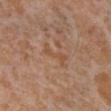The lesion is located on the leg. A female patient aged 53 to 57. Cropped from a whole-body photographic skin survey; the tile spans about 15 mm. Automated tile analysis of the lesion measured a lesion area of about 4 mm², a shape eccentricity near 0.9, and a shape-asymmetry score of about 0.4 (0 = symmetric). The software also gave a mean CIELAB color near L≈51 a*≈20 b*≈33, a lesion–skin lightness drop of about 5, and a normalized lesion–skin contrast near 5.5. It also reported border irregularity of about 6 on a 0–10 scale and radial color variation of about 0. The analysis additionally found a nevus-likeness score of about 0/100 and a lesion-detection confidence of about 100/100. Measured at roughly 3.5 mm in maximum diameter. Imaged with white-light lighting.